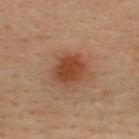The lesion was photographed on a routine skin check and not biopsied; there is no pathology result. A roughly 15 mm field-of-view crop from a total-body skin photograph. The tile uses cross-polarized illumination. Automated image analysis of the tile measured a lesion area of about 9 mm² and a symmetry-axis asymmetry near 0.2. And it measured a border-irregularity index near 2/10, internal color variation of about 2.5 on a 0–10 scale, and radial color variation of about 1. Located on the back. Measured at roughly 4 mm in maximum diameter. A male subject, about 35 years old.